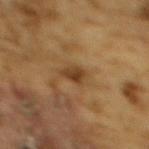Part of a total-body skin-imaging series; this lesion was reviewed on a skin check and was not flagged for biopsy. From the upper back. Cropped from a whole-body photographic skin survey; the tile spans about 15 mm. A male patient, in their mid- to late 80s or older. Imaged with cross-polarized lighting.